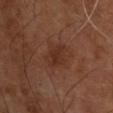Part of a total-body skin-imaging series; this lesion was reviewed on a skin check and was not flagged for biopsy.